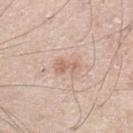The lesion was photographed on a routine skin check and not biopsied; there is no pathology result. A lesion tile, about 15 mm wide, cut from a 3D total-body photograph. Approximately 3 mm at its widest. A male patient, aged approximately 60. Located on the left lower leg.